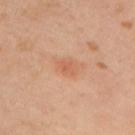Assessment: This lesion was catalogued during total-body skin photography and was not selected for biopsy. Background: A close-up tile cropped from a whole-body skin photograph, about 15 mm across. The lesion's longest dimension is about 2.5 mm. The lesion is on the upper back. Captured under cross-polarized illumination. The patient is a female roughly 40 years of age.